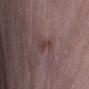This lesion was catalogued during total-body skin photography and was not selected for biopsy. This image is a 15 mm lesion crop taken from a total-body photograph. The patient is a female aged approximately 65. The lesion is on the right lower leg.